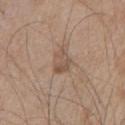Notes:
* body site — the chest
* image source — 15 mm crop, total-body photography
* subject — male, aged 63 to 67
* diameter — ~3 mm (longest diameter)
* lighting — white-light illumination